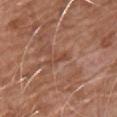{"biopsy_status": "not biopsied; imaged during a skin examination", "patient": {"sex": "male", "age_approx": 60}, "lesion_size": {"long_diameter_mm_approx": 3.0}, "site": "upper back", "lighting": "white-light", "image": {"source": "total-body photography crop", "field_of_view_mm": 15}}